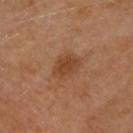<record>
<biopsy_status>not biopsied; imaged during a skin examination</biopsy_status>
<site>head or neck</site>
<patient>
  <sex>female</sex>
  <age_approx>60</age_approx>
</patient>
<lighting>cross-polarized</lighting>
<lesion_size>
  <long_diameter_mm_approx>4.0</long_diameter_mm_approx>
</lesion_size>
<image>
  <source>total-body photography crop</source>
  <field_of_view_mm>15</field_of_view_mm>
</image>
</record>